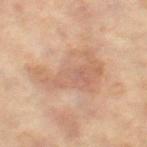Part of a total-body skin-imaging series; this lesion was reviewed on a skin check and was not flagged for biopsy.
A female subject, aged 58–62.
The lesion's longest dimension is about 7.5 mm.
The lesion-visualizer software estimated a border-irregularity rating of about 6/10 and a within-lesion color-variation index near 3.5/10.
A roughly 15 mm field-of-view crop from a total-body skin photograph.
Imaged with cross-polarized lighting.
Located on the right thigh.